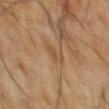Q: Was a biopsy performed?
A: catalogued during a skin exam; not biopsied
Q: Who is the patient?
A: male, about 65 years old
Q: Automated lesion metrics?
A: a lesion area of about 3.5 mm² and a shape eccentricity near 0.9
Q: What is the lesion's diameter?
A: ≈3.5 mm
Q: Lesion location?
A: the chest
Q: Illumination type?
A: cross-polarized
Q: What is the imaging modality?
A: total-body-photography crop, ~15 mm field of view